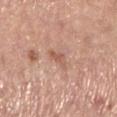Impression:
No biopsy was performed on this lesion — it was imaged during a full skin examination and was not determined to be concerning.
Clinical summary:
The tile uses white-light illumination. From the left lower leg. A female patient aged around 65. About 3 mm across. A 15 mm close-up extracted from a 3D total-body photography capture.